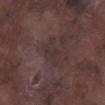This lesion was catalogued during total-body skin photography and was not selected for biopsy.
A roughly 15 mm field-of-view crop from a total-body skin photograph.
A male patient, in their mid- to late 70s.
Imaged with white-light lighting.
The lesion is on the right lower leg.
About 4 mm across.
The total-body-photography lesion software estimated a classifier nevus-likeness of about 0/100.